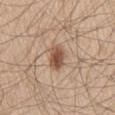Part of a total-body skin-imaging series; this lesion was reviewed on a skin check and was not flagged for biopsy. The lesion is located on the right thigh. This image is a 15 mm lesion crop taken from a total-body photograph. Automated tile analysis of the lesion measured an area of roughly 6 mm², a shape eccentricity near 0.7, and a symmetry-axis asymmetry near 0.15. It also reported a lesion color around L≈52 a*≈19 b*≈30 in CIELAB, roughly 13 lightness units darker than nearby skin, and a normalized border contrast of about 9. The analysis additionally found an automated nevus-likeness rating near 95 out of 100 and a lesion-detection confidence of about 100/100. A male patient aged 58–62.